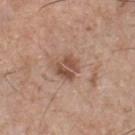This lesion was catalogued during total-body skin photography and was not selected for biopsy. The lesion is on the chest. The lesion's longest dimension is about 3 mm. The lesion-visualizer software estimated an outline eccentricity of about 0.45 (0 = round, 1 = elongated) and two-axis asymmetry of about 0.25. The software also gave a nevus-likeness score of about 50/100 and lesion-presence confidence of about 100/100. Cropped from a whole-body photographic skin survey; the tile spans about 15 mm. The patient is a male in their mid- to late 70s. This is a white-light tile.